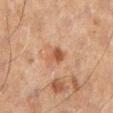Recorded during total-body skin imaging; not selected for excision or biopsy.
A male subject, about 60 years old.
The lesion is on the left lower leg.
A lesion tile, about 15 mm wide, cut from a 3D total-body photograph.
Captured under cross-polarized illumination.